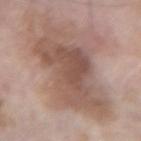follow-up = imaged on a skin check; not biopsied
patient = male, in their 60s
size = about 10.5 mm
automated lesion analysis = an area of roughly 44 mm², a shape eccentricity near 0.8, and two-axis asymmetry of about 0.3; internal color variation of about 5.5 on a 0–10 scale and peripheral color asymmetry of about 1.5; a classifier nevus-likeness of about 10/100 and a detector confidence of about 100 out of 100 that the crop contains a lesion
location = the left forearm
lighting = white-light
image = 15 mm crop, total-body photography